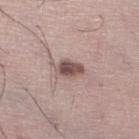Clinical impression:
The lesion was tiled from a total-body skin photograph and was not biopsied.
Acquisition and patient details:
Located on the leg. A 15 mm close-up extracted from a 3D total-body photography capture. A male subject approximately 35 years of age. The tile uses white-light illumination. Longest diameter approximately 3 mm.